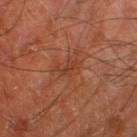The lesion was photographed on a routine skin check and not biopsied; there is no pathology result.
The lesion is located on the right lower leg.
The lesion's longest dimension is about 3 mm.
This is a cross-polarized tile.
A roughly 15 mm field-of-view crop from a total-body skin photograph.
Automated image analysis of the tile measured a lesion area of about 3 mm², an eccentricity of roughly 0.9, and two-axis asymmetry of about 0.55. The software also gave a lesion color around L≈38 a*≈24 b*≈30 in CIELAB and a lesion-to-skin contrast of about 5 (normalized; higher = more distinct). The analysis additionally found a border-irregularity rating of about 6/10 and radial color variation of about 0.
A male patient aged around 65.